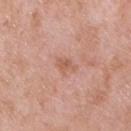The lesion was tiled from a total-body skin photograph and was not biopsied.
From the upper back.
The lesion's longest dimension is about 3 mm.
A 15 mm close-up tile from a total-body photography series done for melanoma screening.
A male patient, in their 50s.
Captured under white-light illumination.